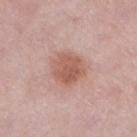biopsy status: imaged on a skin check; not biopsied
site: the left lower leg
image source: total-body-photography crop, ~15 mm field of view
patient: female, aged approximately 40